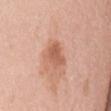The lesion was tiled from a total-body skin photograph and was not biopsied.
Approximately 3.5 mm at its widest.
Automated image analysis of the tile measured a nevus-likeness score of about 5/100 and a lesion-detection confidence of about 100/100.
Located on the front of the torso.
A female subject, aged 63–67.
Cropped from a whole-body photographic skin survey; the tile spans about 15 mm.